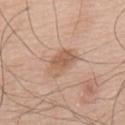Assessment:
Captured during whole-body skin photography for melanoma surveillance; the lesion was not biopsied.
Clinical summary:
Captured under white-light illumination. The recorded lesion diameter is about 4 mm. The lesion is on the upper back. A male subject, about 75 years old. An algorithmic analysis of the crop reported a lesion color around L≈58 a*≈19 b*≈31 in CIELAB and roughly 9 lightness units darker than nearby skin. The software also gave an automated nevus-likeness rating near 0 out of 100. A 15 mm close-up tile from a total-body photography series done for melanoma screening.